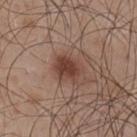tile lighting — white-light
body site — the upper back
lesion size — ≈3.5 mm
acquisition — 15 mm crop, total-body photography
TBP lesion metrics — a lesion color around L≈41 a*≈21 b*≈25 in CIELAB and roughly 11 lightness units darker than nearby skin; a border-irregularity rating of about 1.5/10 and a color-variation rating of about 3.5/10
subject — male, aged approximately 50